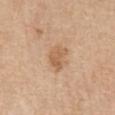biopsy status: total-body-photography surveillance lesion; no biopsy
image-analysis metrics: a lesion–skin lightness drop of about 9 and a lesion-to-skin contrast of about 6.5 (normalized; higher = more distinct); a border-irregularity index near 3/10 and radial color variation of about 1; a lesion-detection confidence of about 100/100
site: the chest
tile lighting: white-light
image source: total-body-photography crop, ~15 mm field of view
size: about 3.5 mm
patient: female, roughly 45 years of age